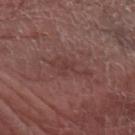Clinical summary: A male patient aged approximately 70. The total-body-photography lesion software estimated an eccentricity of roughly 0.95 and two-axis asymmetry of about 0.55. And it measured an average lesion color of about L≈38 a*≈22 b*≈20 (CIELAB) and a lesion–skin lightness drop of about 6. It also reported an automated nevus-likeness rating near 0 out of 100. A close-up tile cropped from a whole-body skin photograph, about 15 mm across. On the arm. The lesion's longest dimension is about 5 mm. Imaged with white-light lighting.